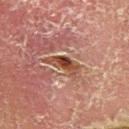<lesion>
<automated_metrics>
  <vs_skin_darker_L>8.0</vs_skin_darker_L>
  <vs_skin_contrast_norm>9.0</vs_skin_contrast_norm>
  <lesion_detection_confidence_0_100>85</lesion_detection_confidence_0_100>
</automated_metrics>
<lighting>cross-polarized</lighting>
<patient>
  <sex>male</sex>
  <age_approx>80</age_approx>
</patient>
<site>left lower leg</site>
<image>
  <source>total-body photography crop</source>
  <field_of_view_mm>15</field_of_view_mm>
</image>
</lesion>